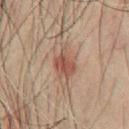Clinical impression:
Imaged during a routine full-body skin examination; the lesion was not biopsied and no histopathology is available.
Clinical summary:
A male subject aged 58–62. Captured under cross-polarized illumination. A 15 mm close-up extracted from a 3D total-body photography capture. Measured at roughly 3.5 mm in maximum diameter. Located on the chest. Automated tile analysis of the lesion measured an area of roughly 7 mm² and a shape eccentricity near 0.65. The software also gave a normalized border contrast of about 7.5. It also reported a border-irregularity rating of about 2.5/10 and internal color variation of about 4 on a 0–10 scale.